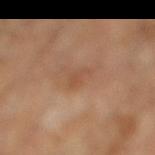follow-up — catalogued during a skin exam; not biopsied
imaging modality — ~15 mm crop, total-body skin-cancer survey
location — the leg
patient — aged around 55